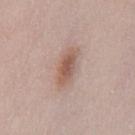Captured during whole-body skin photography for melanoma surveillance; the lesion was not biopsied.
Measured at roughly 4.5 mm in maximum diameter.
A female subject roughly 30 years of age.
This is a white-light tile.
Located on the front of the torso.
A roughly 15 mm field-of-view crop from a total-body skin photograph.